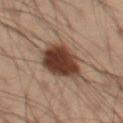Q: Is there a histopathology result?
A: catalogued during a skin exam; not biopsied
Q: Automated lesion metrics?
A: an area of roughly 18 mm², a shape eccentricity near 0.65, and two-axis asymmetry of about 0.2; an average lesion color of about L≈31 a*≈15 b*≈22 (CIELAB), about 14 CIELAB-L* units darker than the surrounding skin, and a lesion-to-skin contrast of about 13 (normalized; higher = more distinct); a border-irregularity index near 2/10, internal color variation of about 4.5 on a 0–10 scale, and radial color variation of about 1.5; an automated nevus-likeness rating near 100 out of 100
Q: What are the patient's age and sex?
A: male, in their mid- to late 50s
Q: What lighting was used for the tile?
A: cross-polarized
Q: What is the imaging modality?
A: ~15 mm tile from a whole-body skin photo
Q: What is the anatomic site?
A: the leg
Q: How large is the lesion?
A: ≈5.5 mm